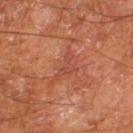workup = catalogued during a skin exam; not biopsied | body site = the right thigh | subject = male, in their mid- to late 60s | imaging modality = total-body-photography crop, ~15 mm field of view.